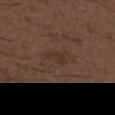This lesion was catalogued during total-body skin photography and was not selected for biopsy.
The patient is a male about 50 years old.
On the upper back.
This image is a 15 mm lesion crop taken from a total-body photograph.